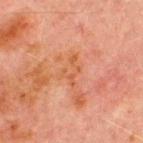The lesion was photographed on a routine skin check and not biopsied; there is no pathology result. On the chest. Imaged with cross-polarized lighting. The lesion's longest dimension is about 3.5 mm. A roughly 15 mm field-of-view crop from a total-body skin photograph. The patient is a male about 70 years old.